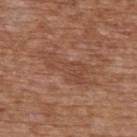Q: Is there a histopathology result?
A: catalogued during a skin exam; not biopsied
Q: Who is the patient?
A: male, aged 73–77
Q: Where on the body is the lesion?
A: the back
Q: How was this image acquired?
A: 15 mm crop, total-body photography